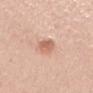Recorded during total-body skin imaging; not selected for excision or biopsy. Imaged with white-light lighting. About 3.5 mm across. A lesion tile, about 15 mm wide, cut from a 3D total-body photograph. A male patient, aged around 35. The lesion is located on the mid back.